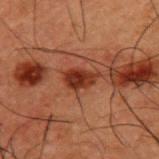Impression:
Part of a total-body skin-imaging series; this lesion was reviewed on a skin check and was not flagged for biopsy.
Acquisition and patient details:
A male patient roughly 50 years of age. Automated tile analysis of the lesion measured an average lesion color of about L≈25 a*≈23 b*≈26 (CIELAB), roughly 10 lightness units darker than nearby skin, and a normalized lesion–skin contrast near 10.5. The analysis additionally found a nevus-likeness score of about 95/100 and a lesion-detection confidence of about 100/100. The lesion's longest dimension is about 3.5 mm. The lesion is located on the back. A lesion tile, about 15 mm wide, cut from a 3D total-body photograph. Captured under cross-polarized illumination.